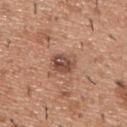| key | value |
|---|---|
| follow-up | imaged on a skin check; not biopsied |
| image source | 15 mm crop, total-body photography |
| lesion size | ~3 mm (longest diameter) |
| tile lighting | white-light illumination |
| location | the upper back |
| automated lesion analysis | a lesion color around L≈49 a*≈21 b*≈27 in CIELAB, a lesion–skin lightness drop of about 12, and a normalized lesion–skin contrast near 9; a peripheral color-asymmetry measure near 2 |
| subject | male, in their 40s |